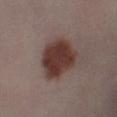  biopsy_status: not biopsied; imaged during a skin examination